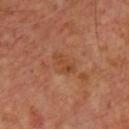Assessment:
Part of a total-body skin-imaging series; this lesion was reviewed on a skin check and was not flagged for biopsy.
Image and clinical context:
A close-up tile cropped from a whole-body skin photograph, about 15 mm across. An algorithmic analysis of the crop reported an outline eccentricity of about 0.9 (0 = round, 1 = elongated) and a symmetry-axis asymmetry near 0.4. And it measured a lesion-detection confidence of about 100/100. Imaged with cross-polarized lighting. Measured at roughly 3 mm in maximum diameter. A male subject, in their mid- to late 60s.